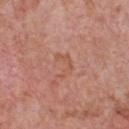Notes:
- follow-up · catalogued during a skin exam; not biopsied
- automated lesion analysis · a footprint of about 4 mm² and a shape-asymmetry score of about 0.6 (0 = symmetric); lesion-presence confidence of about 100/100
- lesion size · about 3 mm
- illumination · white-light
- acquisition · ~15 mm crop, total-body skin-cancer survey
- site · the chest
- subject · male, aged 58–62